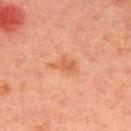Case summary:
* workup · total-body-photography surveillance lesion; no biopsy
* patient · male, approximately 45 years of age
* image source · ~15 mm crop, total-body skin-cancer survey
* size · about 3.5 mm
* site · the mid back